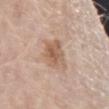| feature | finding |
|---|---|
| workup | imaged on a skin check; not biopsied |
| patient | female, roughly 70 years of age |
| image source | ~15 mm crop, total-body skin-cancer survey |
| lesion size | about 4.5 mm |
| lighting | white-light |
| anatomic site | the right forearm |